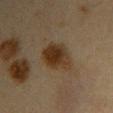Recorded during total-body skin imaging; not selected for excision or biopsy. On the arm. A female patient, aged 43–47. This image is a 15 mm lesion crop taken from a total-body photograph. Captured under cross-polarized illumination. Approximately 5 mm at its widest. The lesion-visualizer software estimated a footprint of about 11 mm² and a symmetry-axis asymmetry near 0.2. It also reported internal color variation of about 4.5 on a 0–10 scale.